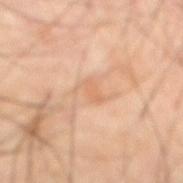Assessment: The lesion was photographed on a routine skin check and not biopsied; there is no pathology result. Background: A male subject, aged approximately 65. From the abdomen. Automated tile analysis of the lesion measured a mean CIELAB color near L≈63 a*≈20 b*≈34, a lesion–skin lightness drop of about 6, and a normalized lesion–skin contrast near 4.5. And it measured a classifier nevus-likeness of about 0/100 and a detector confidence of about 100 out of 100 that the crop contains a lesion. A roughly 15 mm field-of-view crop from a total-body skin photograph. Approximately 3 mm at its widest. The tile uses cross-polarized illumination.